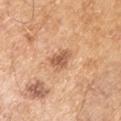The lesion was photographed on a routine skin check and not biopsied; there is no pathology result. The lesion's longest dimension is about 3.5 mm. A close-up tile cropped from a whole-body skin photograph, about 15 mm across. A male subject, aged approximately 55. Located on the left upper arm.